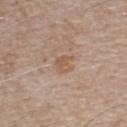lesion size: ≈2.5 mm
image source: total-body-photography crop, ~15 mm field of view
tile lighting: white-light illumination
image-analysis metrics: a footprint of about 4 mm², an eccentricity of roughly 0.5, and a symmetry-axis asymmetry near 0.4; about 7 CIELAB-L* units darker than the surrounding skin; a peripheral color-asymmetry measure near 1
site: the front of the torso
subject: male, about 65 years old